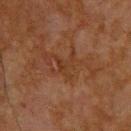notes = catalogued during a skin exam; not biopsied | size = about 3 mm | lighting = cross-polarized illumination | automated metrics = an area of roughly 4 mm², a shape eccentricity near 0.55, and a symmetry-axis asymmetry near 0.7; a border-irregularity index near 9/10, a color-variation rating of about 0.5/10, and peripheral color asymmetry of about 0 | site = the upper back | image = ~15 mm crop, total-body skin-cancer survey | patient = male, aged around 65.